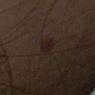Captured during whole-body skin photography for melanoma surveillance; the lesion was not biopsied. A male patient roughly 65 years of age. A 15 mm crop from a total-body photograph taken for skin-cancer surveillance. Longest diameter approximately 2.5 mm. Located on the left upper arm. Captured under cross-polarized illumination.